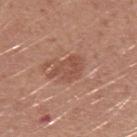Q: Was a biopsy performed?
A: no biopsy performed (imaged during a skin exam)
Q: Where on the body is the lesion?
A: the arm
Q: What is the imaging modality?
A: ~15 mm crop, total-body skin-cancer survey
Q: What are the patient's age and sex?
A: male, approximately 25 years of age
Q: Illumination type?
A: white-light illumination
Q: Lesion size?
A: about 4 mm